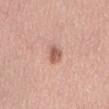Q: Was this lesion biopsied?
A: catalogued during a skin exam; not biopsied
Q: What is the imaging modality?
A: 15 mm crop, total-body photography
Q: Who is the patient?
A: female, aged approximately 65
Q: Lesion location?
A: the abdomen